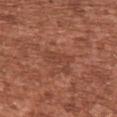biopsy_status: not biopsied; imaged during a skin examination
lighting: white-light
automated_metrics:
  cielab_L: 43
  cielab_a: 25
  cielab_b: 29
  vs_skin_darker_L: 6.0
  vs_skin_contrast_norm: 5.0
site: chest
patient:
  sex: male
  age_approx: 45
image:
  source: total-body photography crop
  field_of_view_mm: 15
lesion_size:
  long_diameter_mm_approx: 3.5